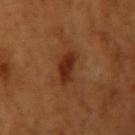Clinical summary: The total-body-photography lesion software estimated an eccentricity of roughly 0.8. The software also gave a lesion color around L≈32 a*≈25 b*≈33 in CIELAB, about 10 CIELAB-L* units darker than the surrounding skin, and a normalized lesion–skin contrast near 9. A 15 mm close-up tile from a total-body photography series done for melanoma screening. The tile uses cross-polarized illumination. The patient is a female aged 53 to 57. Located on the right upper arm.